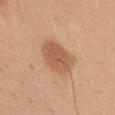| feature | finding |
|---|---|
| biopsy status | imaged on a skin check; not biopsied |
| site | the right upper arm |
| image-analysis metrics | an average lesion color of about L≈57 a*≈23 b*≈34 (CIELAB), roughly 10 lightness units darker than nearby skin, and a normalized border contrast of about 7; a border-irregularity rating of about 1.5/10 and internal color variation of about 3 on a 0–10 scale; a classifier nevus-likeness of about 95/100 and a detector confidence of about 100 out of 100 that the crop contains a lesion |
| lesion size | ~4.5 mm (longest diameter) |
| illumination | white-light illumination |
| imaging modality | total-body-photography crop, ~15 mm field of view |
| patient | female, in their 40s |